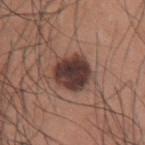Clinical impression: Captured during whole-body skin photography for melanoma surveillance; the lesion was not biopsied. Acquisition and patient details: The total-body-photography lesion software estimated a footprint of about 13 mm², an eccentricity of roughly 0.45, and two-axis asymmetry of about 0.15. The analysis additionally found a mean CIELAB color near L≈36 a*≈18 b*≈21 and roughly 16 lightness units darker than nearby skin. And it measured an automated nevus-likeness rating near 65 out of 100 and lesion-presence confidence of about 100/100. On the arm. A male subject, roughly 35 years of age. A 15 mm close-up extracted from a 3D total-body photography capture. Captured under white-light illumination.